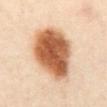Part of a total-body skin-imaging series; this lesion was reviewed on a skin check and was not flagged for biopsy.
The lesion is located on the abdomen.
A 15 mm crop from a total-body photograph taken for skin-cancer surveillance.
A male patient, about 65 years old.